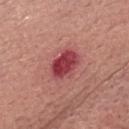Case summary:
• notes — catalogued during a skin exam; not biopsied
• lighting — white-light illumination
• size — ~4 mm (longest diameter)
• body site — the head or neck
• image — 15 mm crop, total-body photography
• patient — male, aged 63 to 67
• automated metrics — a lesion area of about 9.5 mm², an eccentricity of roughly 0.75, and a shape-asymmetry score of about 0.2 (0 = symmetric); a mean CIELAB color near L≈44 a*≈36 b*≈22 and a normalized border contrast of about 10.5; a border-irregularity rating of about 2/10, a color-variation rating of about 4/10, and a peripheral color-asymmetry measure near 1.5; a classifier nevus-likeness of about 0/100 and lesion-presence confidence of about 100/100